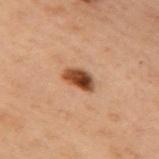Part of a total-body skin-imaging series; this lesion was reviewed on a skin check and was not flagged for biopsy.
The lesion is located on the left arm.
This is a cross-polarized tile.
A 15 mm close-up tile from a total-body photography series done for melanoma screening.
Longest diameter approximately 3.5 mm.
A female patient, in their mid-50s.